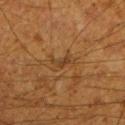| key | value |
|---|---|
| follow-up | imaged on a skin check; not biopsied |
| imaging modality | 15 mm crop, total-body photography |
| lighting | cross-polarized illumination |
| subject | male, aged 58 to 62 |
| body site | the left lower leg |
| size | ≈3.5 mm |
| image-analysis metrics | an area of roughly 4 mm², a shape eccentricity near 0.9, and a symmetry-axis asymmetry near 0.45; a normalized lesion–skin contrast near 5.5; internal color variation of about 0.5 on a 0–10 scale and radial color variation of about 0 |